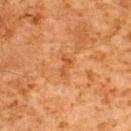Clinical impression:
Part of a total-body skin-imaging series; this lesion was reviewed on a skin check and was not flagged for biopsy.
Context:
A roughly 15 mm field-of-view crop from a total-body skin photograph. The lesion-visualizer software estimated a lesion area of about 2.5 mm², an eccentricity of roughly 0.9, and a symmetry-axis asymmetry near 0.45. It also reported a lesion color around L≈45 a*≈25 b*≈38 in CIELAB and a lesion-to-skin contrast of about 5.5 (normalized; higher = more distinct). The analysis additionally found border irregularity of about 5 on a 0–10 scale, a color-variation rating of about 0/10, and peripheral color asymmetry of about 0. The analysis additionally found a lesion-detection confidence of about 100/100. On the upper back. A male patient about 60 years old.